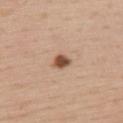Q: Is there a histopathology result?
A: no biopsy performed (imaged during a skin exam)
Q: What is the imaging modality?
A: total-body-photography crop, ~15 mm field of view
Q: Who is the patient?
A: male, roughly 55 years of age
Q: What is the anatomic site?
A: the upper back